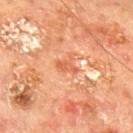{"biopsy_status": "not biopsied; imaged during a skin examination", "patient": {"sex": "male", "age_approx": 65}, "image": {"source": "total-body photography crop", "field_of_view_mm": 15}, "lighting": "cross-polarized", "site": "mid back"}